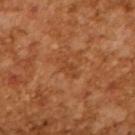follow-up: total-body-photography surveillance lesion; no biopsy | imaging modality: ~15 mm tile from a whole-body skin photo | subject: male, approximately 65 years of age | illumination: cross-polarized illumination.